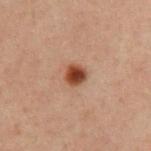Q: Was this lesion biopsied?
A: catalogued during a skin exam; not biopsied
Q: What lighting was used for the tile?
A: cross-polarized
Q: How was this image acquired?
A: ~15 mm tile from a whole-body skin photo
Q: Who is the patient?
A: male, in their 60s
Q: Lesion location?
A: the chest
Q: What is the lesion's diameter?
A: ~2.5 mm (longest diameter)